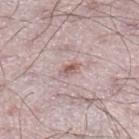| key | value |
|---|---|
| workup | total-body-photography surveillance lesion; no biopsy |
| imaging modality | ~15 mm crop, total-body skin-cancer survey |
| subject | male, about 25 years old |
| tile lighting | white-light illumination |
| anatomic site | the right lower leg |
| size | ~2.5 mm (longest diameter) |